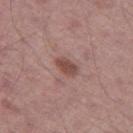No biopsy was performed on this lesion — it was imaged during a full skin examination and was not determined to be concerning. Longest diameter approximately 3 mm. The lesion is located on the left thigh. The patient is a male in their mid- to late 60s. A 15 mm close-up extracted from a 3D total-body photography capture.